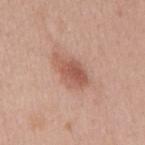{
  "biopsy_status": "not biopsied; imaged during a skin examination",
  "automated_metrics": {
    "area_mm2_approx": 8.5,
    "eccentricity": 0.85,
    "shape_asymmetry": 0.3,
    "cielab_L": 56,
    "cielab_a": 24,
    "cielab_b": 29,
    "vs_skin_darker_L": 11.0,
    "border_irregularity_0_10": 3.5
  },
  "patient": {
    "sex": "female",
    "age_approx": 40
  },
  "image": {
    "source": "total-body photography crop",
    "field_of_view_mm": 15
  },
  "lighting": "white-light",
  "lesion_size": {
    "long_diameter_mm_approx": 4.5
  },
  "site": "mid back"
}